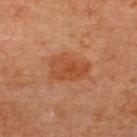No biopsy was performed on this lesion — it was imaged during a full skin examination and was not determined to be concerning. Longest diameter approximately 5 mm. The lesion is located on the upper back. A region of skin cropped from a whole-body photographic capture, roughly 15 mm wide. An algorithmic analysis of the crop reported an area of roughly 12 mm², an eccentricity of roughly 0.8, and a symmetry-axis asymmetry near 0.25. And it measured a nevus-likeness score of about 85/100 and a lesion-detection confidence of about 100/100. A female patient, aged around 65. Captured under cross-polarized illumination.